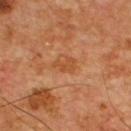follow-up = imaged on a skin check; not biopsied
diameter = ≈2.5 mm
lighting = cross-polarized
patient = male, aged approximately 55
image = ~15 mm tile from a whole-body skin photo
anatomic site = the chest